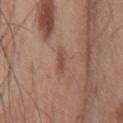A roughly 15 mm field-of-view crop from a total-body skin photograph. The tile uses white-light illumination. The lesion's longest dimension is about 2.5 mm. The patient is a male in their mid-50s. The lesion is on the front of the torso. Automated tile analysis of the lesion measured an area of roughly 3 mm², an eccentricity of roughly 0.9, and a symmetry-axis asymmetry near 0.25. It also reported an average lesion color of about L≈49 a*≈20 b*≈28 (CIELAB), roughly 8 lightness units darker than nearby skin, and a normalized border contrast of about 6. And it measured border irregularity of about 2.5 on a 0–10 scale, internal color variation of about 1 on a 0–10 scale, and a peripheral color-asymmetry measure near 0. It also reported a nevus-likeness score of about 0/100 and lesion-presence confidence of about 100/100.